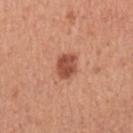biopsy status: catalogued during a skin exam; not biopsied
patient: female, in their 50s
image: ~15 mm crop, total-body skin-cancer survey
lighting: white-light illumination
size: ~3 mm (longest diameter)
location: the left upper arm
TBP lesion metrics: an average lesion color of about L≈51 a*≈28 b*≈32 (CIELAB), roughly 13 lightness units darker than nearby skin, and a lesion-to-skin contrast of about 9 (normalized; higher = more distinct)